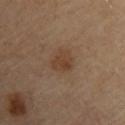Context: About 3 mm across. The total-body-photography lesion software estimated a footprint of about 5 mm², a shape eccentricity near 0.55, and two-axis asymmetry of about 0.3. The software also gave a border-irregularity index near 2.5/10, a color-variation rating of about 2.5/10, and a peripheral color-asymmetry measure near 1. And it measured an automated nevus-likeness rating near 35 out of 100 and a lesion-detection confidence of about 100/100. A male subject, approximately 85 years of age or older. A region of skin cropped from a whole-body photographic capture, roughly 15 mm wide. The lesion is located on the upper back.